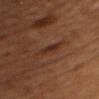The lesion was photographed on a routine skin check and not biopsied; there is no pathology result. The lesion is located on the head or neck. The patient is a male aged 53–57. A roughly 15 mm field-of-view crop from a total-body skin photograph. Automated image analysis of the tile measured a lesion color around L≈29 a*≈21 b*≈26 in CIELAB, about 7 CIELAB-L* units darker than the surrounding skin, and a lesion-to-skin contrast of about 7.5 (normalized; higher = more distinct). And it measured a border-irregularity index near 2.5/10 and peripheral color asymmetry of about 0. The analysis additionally found a lesion-detection confidence of about 75/100. Captured under cross-polarized illumination.